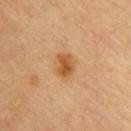| field | value |
|---|---|
| workup | total-body-photography surveillance lesion; no biopsy |
| image source | 15 mm crop, total-body photography |
| location | the upper back |
| size | about 3 mm |
| illumination | cross-polarized illumination |
| patient | female, aged 63 to 67 |